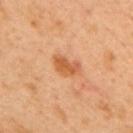biopsy status = no biopsy performed (imaged during a skin exam)
imaging modality = 15 mm crop, total-body photography
subject = male, aged around 50
illumination = cross-polarized illumination
diameter = ≈3.5 mm
anatomic site = the mid back